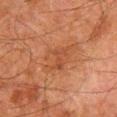Q: Was a biopsy performed?
A: no biopsy performed (imaged during a skin exam)
Q: What are the patient's age and sex?
A: male, roughly 80 years of age
Q: What did automated image analysis measure?
A: a border-irregularity rating of about 4/10, a within-lesion color-variation index near 3/10, and peripheral color asymmetry of about 1
Q: How large is the lesion?
A: ~4.5 mm (longest diameter)
Q: How was the tile lit?
A: cross-polarized illumination
Q: Where on the body is the lesion?
A: the left thigh
Q: What kind of image is this?
A: ~15 mm crop, total-body skin-cancer survey